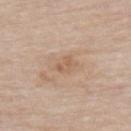No biopsy was performed on this lesion — it was imaged during a full skin examination and was not determined to be concerning.
A male patient, aged 83 or older.
Cropped from a total-body skin-imaging series; the visible field is about 15 mm.
Imaged with white-light lighting.
On the upper back.
About 3 mm across.